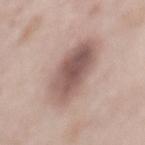Captured during whole-body skin photography for melanoma surveillance; the lesion was not biopsied.
A male patient, approximately 55 years of age.
Imaged with white-light lighting.
Located on the back.
The lesion's longest dimension is about 7 mm.
A 15 mm close-up tile from a total-body photography series done for melanoma screening.